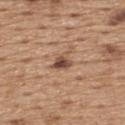Findings:
- biopsy status — catalogued during a skin exam; not biopsied
- patient — male, aged approximately 65
- size — about 2.5 mm
- location — the back
- image — ~15 mm crop, total-body skin-cancer survey
- TBP lesion metrics — a lesion area of about 4 mm², a shape eccentricity near 0.55, and a symmetry-axis asymmetry near 0.35; a lesion color around L≈49 a*≈19 b*≈28 in CIELAB and a normalized lesion–skin contrast near 9; a border-irregularity rating of about 3/10 and radial color variation of about 2; a classifier nevus-likeness of about 75/100